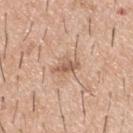Impression:
The lesion was photographed on a routine skin check and not biopsied; there is no pathology result.
Clinical summary:
From the upper back. A male patient aged 38–42. A lesion tile, about 15 mm wide, cut from a 3D total-body photograph.